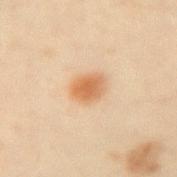Approximately 3 mm at its widest.
A female subject approximately 20 years of age.
The lesion is located on the right forearm.
A close-up tile cropped from a whole-body skin photograph, about 15 mm across.
Captured under cross-polarized illumination.
An algorithmic analysis of the crop reported a footprint of about 6.5 mm², an outline eccentricity of about 0.55 (0 = round, 1 = elongated), and a symmetry-axis asymmetry near 0.2. It also reported an average lesion color of about L≈57 a*≈19 b*≈35 (CIELAB), about 10 CIELAB-L* units darker than the surrounding skin, and a normalized border contrast of about 8. And it measured a border-irregularity rating of about 1.5/10, internal color variation of about 4 on a 0–10 scale, and peripheral color asymmetry of about 1.